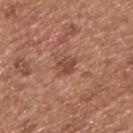workup=no biopsy performed (imaged during a skin exam) | body site=the upper back | patient=male, roughly 55 years of age | illumination=white-light illumination | lesion size=~2.5 mm (longest diameter) | imaging modality=15 mm crop, total-body photography | TBP lesion metrics=roughly 9 lightness units darker than nearby skin and a normalized border contrast of about 7; border irregularity of about 4 on a 0–10 scale, internal color variation of about 2 on a 0–10 scale, and radial color variation of about 0.5.